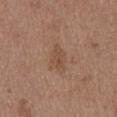The lesion was photographed on a routine skin check and not biopsied; there is no pathology result.
An algorithmic analysis of the crop reported a mean CIELAB color near L≈48 a*≈19 b*≈28, a lesion–skin lightness drop of about 7, and a normalized border contrast of about 5.5.
The lesion is on the abdomen.
The tile uses white-light illumination.
A 15 mm crop from a total-body photograph taken for skin-cancer surveillance.
About 3.5 mm across.
A female subject, aged approximately 65.